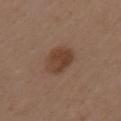biopsy status: total-body-photography surveillance lesion; no biopsy
imaging modality: ~15 mm tile from a whole-body skin photo
size: ~4 mm (longest diameter)
body site: the left upper arm
subject: female, about 40 years old
tile lighting: white-light
image-analysis metrics: a footprint of about 9.5 mm² and a shape eccentricity near 0.7; an average lesion color of about L≈41 a*≈19 b*≈28 (CIELAB), a lesion–skin lightness drop of about 9, and a normalized border contrast of about 8; border irregularity of about 2 on a 0–10 scale, a color-variation rating of about 3.5/10, and a peripheral color-asymmetry measure near 1